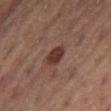notes: imaged on a skin check; not biopsied
anatomic site: the right thigh
illumination: cross-polarized illumination
image: 15 mm crop, total-body photography
size: ~3 mm (longest diameter)
subject: male, approximately 55 years of age